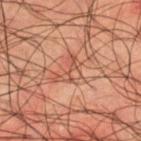Located on the right thigh. A 15 mm crop from a total-body photograph taken for skin-cancer surveillance. The subject is a male about 50 years old.